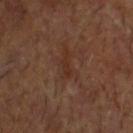Assessment:
The lesion was photographed on a routine skin check and not biopsied; there is no pathology result.
Acquisition and patient details:
A 15 mm close-up extracted from a 3D total-body photography capture. The lesion-visualizer software estimated a footprint of about 2.5 mm², an outline eccentricity of about 0.9 (0 = round, 1 = elongated), and a symmetry-axis asymmetry near 0.6. The lesion is on the head or neck. About 3 mm across. Captured under cross-polarized illumination. The subject is a male about 60 years old.